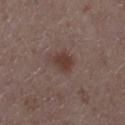Impression:
This lesion was catalogued during total-body skin photography and was not selected for biopsy.
Image and clinical context:
A 15 mm close-up extracted from a 3D total-body photography capture. The lesion is located on the right lower leg. A female patient about 40 years old.